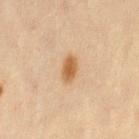Case summary:
– biopsy status — catalogued during a skin exam; not biopsied
– TBP lesion metrics — a lesion color around L≈52 a*≈18 b*≈35 in CIELAB, a lesion–skin lightness drop of about 11, and a normalized lesion–skin contrast near 9; internal color variation of about 2 on a 0–10 scale and radial color variation of about 0.5; an automated nevus-likeness rating near 95 out of 100 and lesion-presence confidence of about 100/100
– patient — female, approximately 45 years of age
– lighting — cross-polarized
– image source — 15 mm crop, total-body photography
– body site — the left thigh
– diameter — about 3 mm